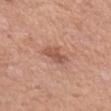Assessment: Imaged during a routine full-body skin examination; the lesion was not biopsied and no histopathology is available. Background: The tile uses white-light illumination. A lesion tile, about 15 mm wide, cut from a 3D total-body photograph. Located on the left upper arm. A female patient aged 58–62.